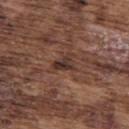{
  "biopsy_status": "not biopsied; imaged during a skin examination",
  "automated_metrics": {
    "cielab_L": 33,
    "cielab_a": 17,
    "cielab_b": 21,
    "vs_skin_darker_L": 10.0,
    "vs_skin_contrast_norm": 9.5,
    "border_irregularity_0_10": 4.0,
    "color_variation_0_10": 4.0,
    "peripheral_color_asymmetry": 1.5,
    "lesion_detection_confidence_0_100": 55
  },
  "lesion_size": {
    "long_diameter_mm_approx": 2.5
  },
  "image": {
    "source": "total-body photography crop",
    "field_of_view_mm": 15
  },
  "lighting": "white-light",
  "site": "upper back",
  "patient": {
    "sex": "male",
    "age_approx": 75
  }
}